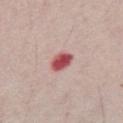Assessment:
The lesion was photographed on a routine skin check and not biopsied; there is no pathology result.
Background:
The lesion is on the abdomen. Imaged with white-light lighting. Approximately 3 mm at its widest. The lesion-visualizer software estimated a lesion area of about 5 mm², an outline eccentricity of about 0.8 (0 = round, 1 = elongated), and a shape-asymmetry score of about 0.15 (0 = symmetric). The analysis additionally found a lesion color around L≈52 a*≈33 b*≈22 in CIELAB and roughly 18 lightness units darker than nearby skin. The software also gave lesion-presence confidence of about 100/100. A male patient, aged 68–72. A lesion tile, about 15 mm wide, cut from a 3D total-body photograph.